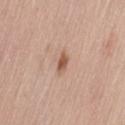Q: Is there a histopathology result?
A: catalogued during a skin exam; not biopsied
Q: What lighting was used for the tile?
A: white-light
Q: Where on the body is the lesion?
A: the left thigh
Q: What are the patient's age and sex?
A: female, about 65 years old
Q: What is the imaging modality?
A: 15 mm crop, total-body photography
Q: Automated lesion metrics?
A: an average lesion color of about L≈56 a*≈21 b*≈30 (CIELAB); border irregularity of about 3.5 on a 0–10 scale and a color-variation rating of about 1.5/10; an automated nevus-likeness rating near 80 out of 100 and lesion-presence confidence of about 100/100